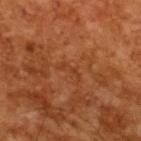{"biopsy_status": "not biopsied; imaged during a skin examination", "patient": {"sex": "male", "age_approx": 65}, "image": {"source": "total-body photography crop", "field_of_view_mm": 15}}